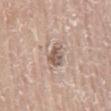Clinical impression:
No biopsy was performed on this lesion — it was imaged during a full skin examination and was not determined to be concerning.
Clinical summary:
The recorded lesion diameter is about 3 mm. The subject is a female aged around 85 or older. Located on the mid back. The tile uses white-light illumination. A region of skin cropped from a whole-body photographic capture, roughly 15 mm wide.